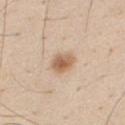Clinical impression:
Imaged during a routine full-body skin examination; the lesion was not biopsied and no histopathology is available.
Context:
The lesion is located on the chest. Cropped from a whole-body photographic skin survey; the tile spans about 15 mm. Captured under white-light illumination. A male subject aged around 30. About 3 mm across.